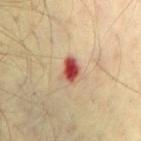Recorded during total-body skin imaging; not selected for excision or biopsy. Located on the chest. A lesion tile, about 15 mm wide, cut from a 3D total-body photograph. The lesion-visualizer software estimated a footprint of about 5 mm² and a symmetry-axis asymmetry near 0.25. It also reported a mean CIELAB color near L≈51 a*≈30 b*≈30 and a normalized lesion–skin contrast near 12. The software also gave a nevus-likeness score of about 0/100 and a detector confidence of about 100 out of 100 that the crop contains a lesion. A male patient, roughly 70 years of age. Captured under cross-polarized illumination.